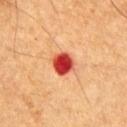The lesion was photographed on a routine skin check and not biopsied; there is no pathology result.
A male patient, aged 63–67.
A 15 mm close-up extracted from a 3D total-body photography capture.
The lesion is located on the chest.
The tile uses cross-polarized illumination.